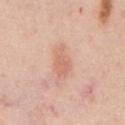Clinical impression:
The lesion was photographed on a routine skin check and not biopsied; there is no pathology result.
Acquisition and patient details:
A male patient aged around 60. The tile uses white-light illumination. A 15 mm crop from a total-body photograph taken for skin-cancer surveillance. Automated tile analysis of the lesion measured a classifier nevus-likeness of about 25/100 and a lesion-detection confidence of about 100/100. The lesion is on the mid back.